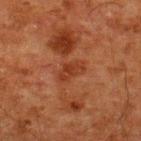Imaged during a routine full-body skin examination; the lesion was not biopsied and no histopathology is available. The lesion is located on the upper back. A male subject aged approximately 60. A 15 mm close-up tile from a total-body photography series done for melanoma screening.